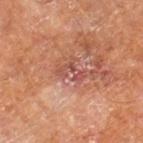This lesion was catalogued during total-body skin photography and was not selected for biopsy. The tile uses cross-polarized illumination. A 15 mm close-up tile from a total-body photography series done for melanoma screening. From the right leg. The subject is a male about 60 years old.